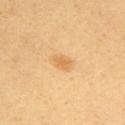Case summary:
* notes · catalogued during a skin exam; not biopsied
* acquisition · total-body-photography crop, ~15 mm field of view
* automated lesion analysis · an area of roughly 5 mm² and a symmetry-axis asymmetry near 0.25; an average lesion color of about L≈70 a*≈20 b*≈46 (CIELAB) and roughly 8 lightness units darker than nearby skin
* patient · female, roughly 40 years of age
* lighting · cross-polarized
* anatomic site · the left arm